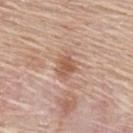The lesion was photographed on a routine skin check and not biopsied; there is no pathology result. The lesion is on the upper back. A region of skin cropped from a whole-body photographic capture, roughly 15 mm wide. The patient is a female aged 63 to 67. About 3 mm across. Captured under white-light illumination.